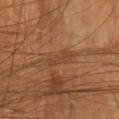The lesion was photographed on a routine skin check and not biopsied; there is no pathology result.
The lesion is located on the head or neck.
The lesion-visualizer software estimated an area of roughly 4 mm² and a symmetry-axis asymmetry near 0.35. The analysis additionally found a mean CIELAB color near L≈44 a*≈20 b*≈34, a lesion–skin lightness drop of about 6, and a lesion-to-skin contrast of about 5 (normalized; higher = more distinct). It also reported a nevus-likeness score of about 0/100 and a lesion-detection confidence of about 70/100.
A female patient, about 60 years old.
This is a cross-polarized tile.
A roughly 15 mm field-of-view crop from a total-body skin photograph.